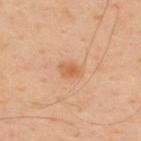{"biopsy_status": "not biopsied; imaged during a skin examination", "automated_metrics": {"vs_skin_darker_L": 8.0, "vs_skin_contrast_norm": 7.0, "nevus_likeness_0_100": 65, "lesion_detection_confidence_0_100": 100}, "lesion_size": {"long_diameter_mm_approx": 2.5}, "lighting": "cross-polarized", "site": "upper back", "image": {"source": "total-body photography crop", "field_of_view_mm": 15}, "patient": {"sex": "male", "age_approx": 45}}